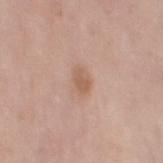Q: Was this lesion biopsied?
A: catalogued during a skin exam; not biopsied
Q: How large is the lesion?
A: ≈2.5 mm
Q: Who is the patient?
A: female, aged 48–52
Q: How was this image acquired?
A: 15 mm crop, total-body photography
Q: Where on the body is the lesion?
A: the left thigh
Q: Automated lesion metrics?
A: a lesion area of about 3.5 mm² and two-axis asymmetry of about 0.3; a mean CIELAB color near L≈58 a*≈20 b*≈29, roughly 9 lightness units darker than nearby skin, and a normalized lesion–skin contrast near 6.5; an automated nevus-likeness rating near 60 out of 100 and lesion-presence confidence of about 100/100
Q: Illumination type?
A: white-light illumination